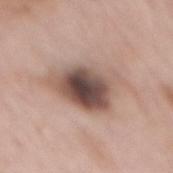Q: Was a biopsy performed?
A: catalogued during a skin exam; not biopsied